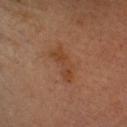The lesion was photographed on a routine skin check and not biopsied; there is no pathology result.
Cropped from a total-body skin-imaging series; the visible field is about 15 mm.
From the head or neck.
A female patient, roughly 30 years of age.
An algorithmic analysis of the crop reported an eccentricity of roughly 0.9 and two-axis asymmetry of about 0.4. The software also gave about 6 CIELAB-L* units darker than the surrounding skin.